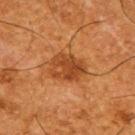The lesion was tiled from a total-body skin photograph and was not biopsied.
The lesion is located on the upper back.
A male subject, aged 63 to 67.
A 15 mm crop from a total-body photograph taken for skin-cancer surveillance.
Approximately 4.5 mm at its widest.